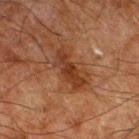Notes:
• biopsy status · catalogued during a skin exam; not biopsied
• lighting · cross-polarized
• subject · male, aged around 80
• body site · the right thigh
• imaging modality · ~15 mm crop, total-body skin-cancer survey
• size · ~5 mm (longest diameter)